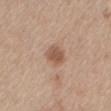{"biopsy_status": "not biopsied; imaged during a skin examination", "site": "back", "lesion_size": {"long_diameter_mm_approx": 3.0}, "patient": {"sex": "male", "age_approx": 65}, "image": {"source": "total-body photography crop", "field_of_view_mm": 15}}